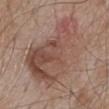notes = no biopsy performed (imaged during a skin exam).